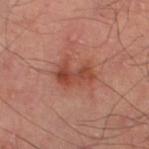image = 15 mm crop, total-body photography | diameter = about 4.5 mm | site = the left thigh.